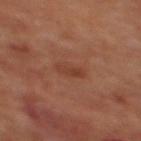follow-up = no biopsy performed (imaged during a skin exam).